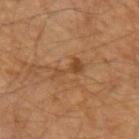Recorded during total-body skin imaging; not selected for excision or biopsy. A 15 mm close-up extracted from a 3D total-body photography capture. The lesion-visualizer software estimated an average lesion color of about L≈44 a*≈21 b*≈34 (CIELAB), a lesion–skin lightness drop of about 8, and a lesion-to-skin contrast of about 6.5 (normalized; higher = more distinct). The analysis additionally found an automated nevus-likeness rating near 5 out of 100 and lesion-presence confidence of about 100/100. Located on the left forearm. The lesion's longest dimension is about 4 mm. A male patient aged approximately 55.